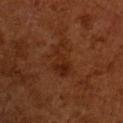No biopsy was performed on this lesion — it was imaged during a full skin examination and was not determined to be concerning. Cropped from a whole-body photographic skin survey; the tile spans about 15 mm. An algorithmic analysis of the crop reported border irregularity of about 6.5 on a 0–10 scale, a color-variation rating of about 2.5/10, and a peripheral color-asymmetry measure near 1. Measured at roughly 4 mm in maximum diameter. A male subject aged 63–67.